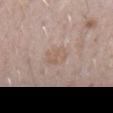The lesion was photographed on a routine skin check and not biopsied; there is no pathology result.
A lesion tile, about 15 mm wide, cut from a 3D total-body photograph.
The patient is a male aged 28–32.
Located on the arm.
The lesion's longest dimension is about 3 mm.